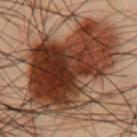| field | value |
|---|---|
| workup | total-body-photography surveillance lesion; no biopsy |
| illumination | cross-polarized illumination |
| imaging modality | ~15 mm crop, total-body skin-cancer survey |
| automated lesion analysis | a lesion area of about 75 mm², a shape eccentricity near 0.8, and a symmetry-axis asymmetry near 0.3; an automated nevus-likeness rating near 65 out of 100 and lesion-presence confidence of about 100/100 |
| lesion diameter | ~13 mm (longest diameter) |
| patient | male, about 55 years old |
| site | the chest |